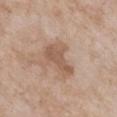This lesion was catalogued during total-body skin photography and was not selected for biopsy. A female subject, approximately 75 years of age. From the chest. The lesion's longest dimension is about 4.5 mm. The total-body-photography lesion software estimated two-axis asymmetry of about 0.5. The analysis additionally found a mean CIELAB color near L≈56 a*≈18 b*≈29, roughly 9 lightness units darker than nearby skin, and a normalized border contrast of about 6.5. The software also gave an automated nevus-likeness rating near 5 out of 100 and a detector confidence of about 100 out of 100 that the crop contains a lesion. This image is a 15 mm lesion crop taken from a total-body photograph.